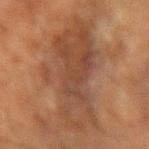A male subject aged approximately 60. On the arm. A 15 mm close-up tile from a total-body photography series done for melanoma screening.